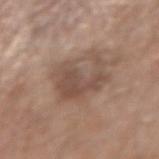  biopsy_status: not biopsied; imaged during a skin examination
  patient:
    sex: male
    age_approx: 65
  lesion_size:
    long_diameter_mm_approx: 5.0
  lighting: white-light
  site: right forearm
  image:
    source: total-body photography crop
    field_of_view_mm: 15
  automated_metrics:
    area_mm2_approx: 15.0
    eccentricity: 0.55
    shape_asymmetry: 0.55
    vs_skin_contrast_norm: 6.5